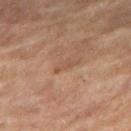Findings:
- subject — female, aged 68 to 72
- anatomic site — the right thigh
- acquisition — ~15 mm tile from a whole-body skin photo